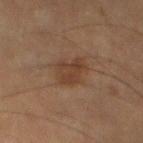This is a cross-polarized tile.
A male subject aged 83 or over.
Longest diameter approximately 4 mm.
A 15 mm close-up tile from a total-body photography series done for melanoma screening.
From the left thigh.